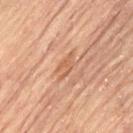workup = imaged on a skin check; not biopsied | body site = the lower back | diameter = ~3 mm (longest diameter) | acquisition = 15 mm crop, total-body photography | subject = female, about 80 years old.